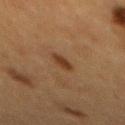automated lesion analysis=a border-irregularity rating of about 3/10, internal color variation of about 1.5 on a 0–10 scale, and peripheral color asymmetry of about 0.5; a lesion-detection confidence of about 100/100
location=the mid back
subject=female, aged 53–57
acquisition=~15 mm tile from a whole-body skin photo
diameter=~2.5 mm (longest diameter)
illumination=cross-polarized illumination